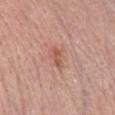  biopsy_status: not biopsied; imaged during a skin examination
  automated_metrics:
    area_mm2_approx: 3.0
    eccentricity: 0.9
    color_variation_0_10: 1.0
  lighting: white-light
  patient:
    sex: female
    age_approx: 65
  site: chest
  image:
    source: total-body photography crop
    field_of_view_mm: 15
  lesion_size:
    long_diameter_mm_approx: 3.0